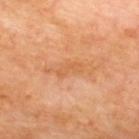{
  "biopsy_status": "not biopsied; imaged during a skin examination",
  "lighting": "cross-polarized",
  "patient": {
    "sex": "male",
    "age_approx": 70
  },
  "site": "back",
  "lesion_size": {
    "long_diameter_mm_approx": 3.5
  },
  "image": {
    "source": "total-body photography crop",
    "field_of_view_mm": 15
  }
}